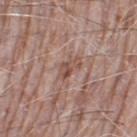<tbp_lesion>
<biopsy_status>not biopsied; imaged during a skin examination</biopsy_status>
<patient>
  <sex>male</sex>
  <age_approx>75</age_approx>
</patient>
<site>left thigh</site>
<image>
  <source>total-body photography crop</source>
  <field_of_view_mm>15</field_of_view_mm>
</image>
</tbp_lesion>